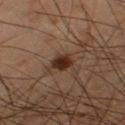Captured during whole-body skin photography for melanoma surveillance; the lesion was not biopsied. The lesion is located on the leg. A male patient aged approximately 60. Captured under cross-polarized illumination. Approximately 2.5 mm at its widest. A 15 mm close-up extracted from a 3D total-body photography capture.